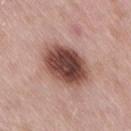Captured during whole-body skin photography for melanoma surveillance; the lesion was not biopsied.